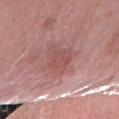A 15 mm close-up tile from a total-body photography series done for melanoma screening. On the right forearm. This is a white-light tile. The subject is a female approximately 65 years of age. Measured at roughly 4.5 mm in maximum diameter.